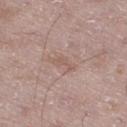biopsy status = catalogued during a skin exam; not biopsied
patient = male, aged around 50
image = 15 mm crop, total-body photography
body site = the right thigh